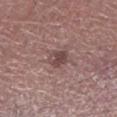workup: no biopsy performed (imaged during a skin exam)
imaging modality: ~15 mm tile from a whole-body skin photo
tile lighting: white-light
patient: male, about 45 years old
automated metrics: a footprint of about 4.5 mm², an outline eccentricity of about 0.3 (0 = round, 1 = elongated), and a shape-asymmetry score of about 0.2 (0 = symmetric); a lesion-to-skin contrast of about 7.5 (normalized; higher = more distinct)
site: the leg